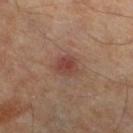notes — catalogued during a skin exam; not biopsied
site — the left lower leg
subject — male, in their mid-50s
tile lighting — cross-polarized
acquisition — total-body-photography crop, ~15 mm field of view
automated lesion analysis — a lesion–skin lightness drop of about 9 and a lesion-to-skin contrast of about 7 (normalized; higher = more distinct); a nevus-likeness score of about 40/100 and a lesion-detection confidence of about 100/100